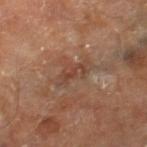Assessment:
Imaged during a routine full-body skin examination; the lesion was not biopsied and no histopathology is available.
Clinical summary:
Measured at roughly 3 mm in maximum diameter. This is a cross-polarized tile. A lesion tile, about 15 mm wide, cut from a 3D total-body photograph. A male patient, aged 68–72. Located on the right thigh.